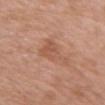No biopsy was performed on this lesion — it was imaged during a full skin examination and was not determined to be concerning. Imaged with white-light lighting. A female subject, about 70 years old. About 5 mm across. An algorithmic analysis of the crop reported a footprint of about 8.5 mm², an outline eccentricity of about 0.85 (0 = round, 1 = elongated), and a symmetry-axis asymmetry near 0.55. The software also gave border irregularity of about 6 on a 0–10 scale, a within-lesion color-variation index near 3/10, and radial color variation of about 1. Located on the front of the torso. A region of skin cropped from a whole-body photographic capture, roughly 15 mm wide.